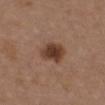Clinical impression:
No biopsy was performed on this lesion — it was imaged during a full skin examination and was not determined to be concerning.
Background:
A 15 mm close-up tile from a total-body photography series done for melanoma screening. On the chest. A female subject about 40 years old.